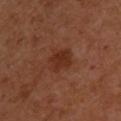Recorded during total-body skin imaging; not selected for excision or biopsy. On the back. A roughly 15 mm field-of-view crop from a total-body skin photograph. An algorithmic analysis of the crop reported a mean CIELAB color near L≈31 a*≈24 b*≈29 and roughly 7 lightness units darker than nearby skin. A female patient, aged 38–42. The lesion's longest dimension is about 3.5 mm. The tile uses cross-polarized illumination.